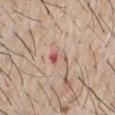biopsy status = catalogued during a skin exam; not biopsied | anatomic site = the chest | lesion diameter = about 2.5 mm | tile lighting = white-light illumination | acquisition = total-body-photography crop, ~15 mm field of view | subject = male, roughly 65 years of age.